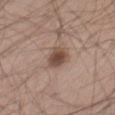{
  "patient": {
    "sex": "male",
    "age_approx": 60
  },
  "site": "right thigh",
  "lesion_size": {
    "long_diameter_mm_approx": 3.0
  },
  "image": {
    "source": "total-body photography crop",
    "field_of_view_mm": 15
  },
  "automated_metrics": {
    "area_mm2_approx": 5.5,
    "eccentricity": 0.65,
    "shape_asymmetry": 0.25,
    "cielab_L": 47,
    "cielab_a": 18,
    "cielab_b": 25,
    "vs_skin_darker_L": 13.0,
    "vs_skin_contrast_norm": 9.5,
    "nevus_likeness_0_100": 95,
    "lesion_detection_confidence_0_100": 100
  }
}